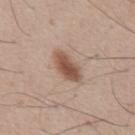Q: Is there a histopathology result?
A: imaged on a skin check; not biopsied
Q: How was this image acquired?
A: 15 mm crop, total-body photography
Q: Who is the patient?
A: male, aged approximately 55